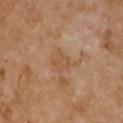| feature | finding |
|---|---|
| follow-up | catalogued during a skin exam; not biopsied |
| automated metrics | an area of roughly 6.5 mm², an outline eccentricity of about 0.65 (0 = round, 1 = elongated), and a shape-asymmetry score of about 0.35 (0 = symmetric); a normalized lesion–skin contrast near 5; border irregularity of about 4 on a 0–10 scale, internal color variation of about 2 on a 0–10 scale, and radial color variation of about 0.5; a nevus-likeness score of about 0/100 |
| subject | female, about 50 years old |
| image | 15 mm crop, total-body photography |
| location | the chest |
| lesion size | about 3.5 mm |